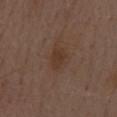  biopsy_status: not biopsied; imaged during a skin examination
  site: mid back
  image:
    source: total-body photography crop
    field_of_view_mm: 15
  patient:
    sex: male
    age_approx: 70
  lesion_size:
    long_diameter_mm_approx: 3.5